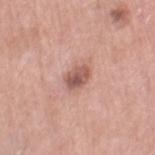workup — no biopsy performed (imaged during a skin exam) | subject — female, about 55 years old | automated lesion analysis — a mean CIELAB color near L≈55 a*≈23 b*≈25 and a lesion–skin lightness drop of about 13; a within-lesion color-variation index near 4.5/10 and a peripheral color-asymmetry measure near 1.5 | illumination — white-light | anatomic site — the right thigh | image — ~15 mm crop, total-body skin-cancer survey.